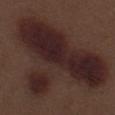Cropped from a whole-body photographic skin survey; the tile spans about 15 mm. Captured under white-light illumination. Approximately 14.5 mm at its widest. Located on the leg. The patient is a male roughly 70 years of age.